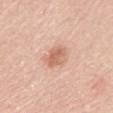biopsy status=total-body-photography surveillance lesion; no biopsy
illumination=white-light
acquisition=15 mm crop, total-body photography
patient=female, approximately 60 years of age
anatomic site=the mid back
size=≈3.5 mm